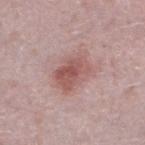Case summary:
• follow-up: no biopsy performed (imaged during a skin exam)
• patient: female, approximately 40 years of age
• image: 15 mm crop, total-body photography
• body site: the left lower leg